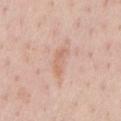The lesion was photographed on a routine skin check and not biopsied; there is no pathology result. The lesion's longest dimension is about 3.5 mm. Located on the chest. A 15 mm close-up extracted from a 3D total-body photography capture. The subject is a male aged approximately 60. This is a white-light tile.